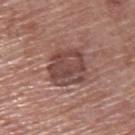The lesion was photographed on a routine skin check and not biopsied; there is no pathology result. The subject is a male roughly 55 years of age. From the upper back. Captured under white-light illumination. A region of skin cropped from a whole-body photographic capture, roughly 15 mm wide. The total-body-photography lesion software estimated an area of roughly 16 mm² and a symmetry-axis asymmetry near 0.15. And it measured border irregularity of about 2 on a 0–10 scale, a within-lesion color-variation index near 4.5/10, and radial color variation of about 1.5.